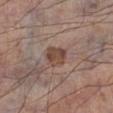Impression:
Recorded during total-body skin imaging; not selected for excision or biopsy.
Context:
The tile uses white-light illumination. The total-body-photography lesion software estimated an area of roughly 6.5 mm² and a shape-asymmetry score of about 0.15 (0 = symmetric). The software also gave an automated nevus-likeness rating near 15 out of 100. The subject is a male approximately 70 years of age. A 15 mm close-up extracted from a 3D total-body photography capture. The recorded lesion diameter is about 3 mm. Located on the right lower leg.